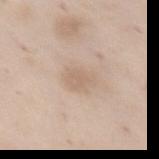subject — female, roughly 55 years of age | image source — total-body-photography crop, ~15 mm field of view | anatomic site — the left thigh.